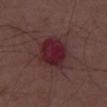Clinical impression:
Imaged during a routine full-body skin examination; the lesion was not biopsied and no histopathology is available.